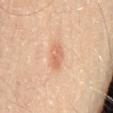| feature | finding |
|---|---|
| patient | male, approximately 65 years of age |
| illumination | cross-polarized illumination |
| body site | the lower back |
| imaging modality | ~15 mm tile from a whole-body skin photo |